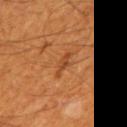{"biopsy_status": "not biopsied; imaged during a skin examination", "image": {"source": "total-body photography crop", "field_of_view_mm": 15}, "patient": {"sex": "male", "age_approx": 60}, "site": "mid back"}